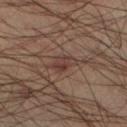Assessment:
This lesion was catalogued during total-body skin photography and was not selected for biopsy.
Background:
A male subject aged 33–37. A close-up tile cropped from a whole-body skin photograph, about 15 mm across. This is a cross-polarized tile. An algorithmic analysis of the crop reported a footprint of about 4.5 mm², an eccentricity of roughly 0.75, and a symmetry-axis asymmetry near 0.3. It also reported a lesion color around L≈34 a*≈16 b*≈21 in CIELAB and a normalized lesion–skin contrast near 6. Approximately 3 mm at its widest. Located on the left lower leg.